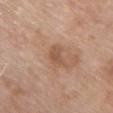This lesion was catalogued during total-body skin photography and was not selected for biopsy. Captured under white-light illumination. The total-body-photography lesion software estimated an area of roughly 4 mm², an outline eccentricity of about 0.7 (0 = round, 1 = elongated), and two-axis asymmetry of about 0.35. The software also gave about 8 CIELAB-L* units darker than the surrounding skin and a lesion-to-skin contrast of about 5.5 (normalized; higher = more distinct). The analysis additionally found a border-irregularity rating of about 3.5/10, a within-lesion color-variation index near 2/10, and radial color variation of about 0.5. The analysis additionally found a nevus-likeness score of about 0/100 and lesion-presence confidence of about 100/100. A close-up tile cropped from a whole-body skin photograph, about 15 mm across. The patient is a female aged 73–77. On the chest.